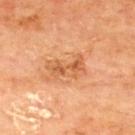Impression:
The lesion was tiled from a total-body skin photograph and was not biopsied.
Clinical summary:
Located on the upper back. A lesion tile, about 15 mm wide, cut from a 3D total-body photograph. Captured under cross-polarized illumination. Automated image analysis of the tile measured a border-irregularity index near 4.5/10, a color-variation rating of about 5/10, and radial color variation of about 1.5. The analysis additionally found an automated nevus-likeness rating near 0 out of 100. The recorded lesion diameter is about 4.5 mm. A male subject about 65 years old.